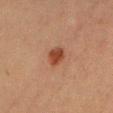Q: Was this lesion biopsied?
A: imaged on a skin check; not biopsied
Q: Patient demographics?
A: male, aged 38 to 42
Q: What kind of image is this?
A: ~15 mm tile from a whole-body skin photo
Q: What is the anatomic site?
A: the chest
Q: Lesion size?
A: about 3 mm
Q: What lighting was used for the tile?
A: cross-polarized illumination
Q: What did automated image analysis measure?
A: an eccentricity of roughly 0.65 and a symmetry-axis asymmetry near 0.15; an average lesion color of about L≈36 a*≈23 b*≈28 (CIELAB), a lesion–skin lightness drop of about 10, and a lesion-to-skin contrast of about 9 (normalized; higher = more distinct); a border-irregularity rating of about 1.5/10, a color-variation rating of about 2.5/10, and peripheral color asymmetry of about 1; a nevus-likeness score of about 100/100 and a lesion-detection confidence of about 100/100